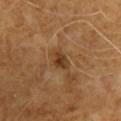The lesion was photographed on a routine skin check and not biopsied; there is no pathology result. A 15 mm close-up tile from a total-body photography series done for melanoma screening. The recorded lesion diameter is about 2.5 mm. The patient is a male aged 58 to 62. From the chest.